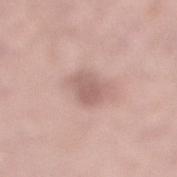  biopsy_status: not biopsied; imaged during a skin examination
  lighting: white-light
  site: leg
  automated_metrics:
    area_mm2_approx: 6.0
    shape_asymmetry: 0.25
    cielab_L: 59
    cielab_a: 20
    cielab_b: 23
    vs_skin_darker_L: 10.0
    vs_skin_contrast_norm: 6.5
  image:
    source: total-body photography crop
    field_of_view_mm: 15
  patient:
    sex: male
    age_approx: 50
  lesion_size:
    long_diameter_mm_approx: 3.5